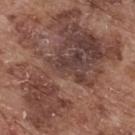Notes:
• notes · imaged on a skin check; not biopsied
• automated lesion analysis · a lesion area of about 65 mm², a shape eccentricity near 0.85, and a shape-asymmetry score of about 0.55 (0 = symmetric); a border-irregularity rating of about 9/10 and a within-lesion color-variation index near 6.5/10; a classifier nevus-likeness of about 0/100
• anatomic site · the upper back
• diameter · about 13.5 mm
• lighting · white-light
• patient · male, aged around 75
• imaging modality · ~15 mm tile from a whole-body skin photo